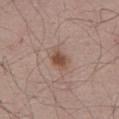workup: total-body-photography surveillance lesion; no biopsy
image-analysis metrics: an area of roughly 5 mm², an outline eccentricity of about 0.65 (0 = round, 1 = elongated), and two-axis asymmetry of about 0.2; a border-irregularity index near 2/10, a color-variation rating of about 3/10, and a peripheral color-asymmetry measure near 1; a nevus-likeness score of about 90/100 and a lesion-detection confidence of about 100/100
illumination: white-light illumination
image: ~15 mm crop, total-body skin-cancer survey
anatomic site: the abdomen
patient: male, aged approximately 60
lesion diameter: about 3 mm